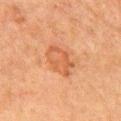Case summary:
* follow-up — catalogued during a skin exam; not biopsied
* patient — male, aged 78–82
* location — the chest
* lighting — cross-polarized
* imaging modality — 15 mm crop, total-body photography